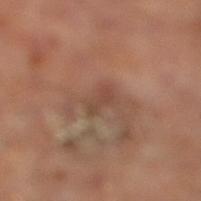Recorded during total-body skin imaging; not selected for excision or biopsy.
About 2.5 mm across.
This is a cross-polarized tile.
The total-body-photography lesion software estimated an outline eccentricity of about 0.9 (0 = round, 1 = elongated) and two-axis asymmetry of about 0.4. It also reported a mean CIELAB color near L≈43 a*≈20 b*≈26 and a lesion–skin lightness drop of about 6.
A male subject aged 63–67.
From the right lower leg.
A 15 mm close-up extracted from a 3D total-body photography capture.